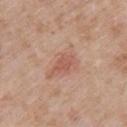body site: the left upper arm | image source: 15 mm crop, total-body photography | patient: female, aged 68–72 | illumination: white-light illumination.